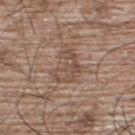Impression: No biopsy was performed on this lesion — it was imaged during a full skin examination and was not determined to be concerning. Acquisition and patient details: Captured under white-light illumination. A region of skin cropped from a whole-body photographic capture, roughly 15 mm wide. A male patient aged 63–67. The lesion is located on the upper back. Approximately 4 mm at its widest. Automated image analysis of the tile measured a lesion area of about 7 mm². And it measured a mean CIELAB color near L≈49 a*≈16 b*≈26, roughly 8 lightness units darker than nearby skin, and a normalized border contrast of about 6. The analysis additionally found border irregularity of about 8 on a 0–10 scale, internal color variation of about 3 on a 0–10 scale, and radial color variation of about 1.